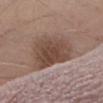follow-up = total-body-photography surveillance lesion; no biopsy
image = 15 mm crop, total-body photography
subject = male, approximately 75 years of age
lesion diameter = about 7 mm
site = the abdomen